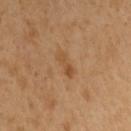Q: Was this lesion biopsied?
A: imaged on a skin check; not biopsied
Q: How was this image acquired?
A: ~15 mm crop, total-body skin-cancer survey
Q: Who is the patient?
A: male, about 50 years old
Q: Lesion size?
A: about 3 mm
Q: What is the anatomic site?
A: the right upper arm